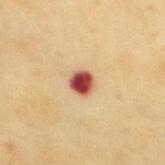This lesion was catalogued during total-body skin photography and was not selected for biopsy. A female subject, approximately 60 years of age. On the mid back. Cropped from a whole-body photographic skin survey; the tile spans about 15 mm.